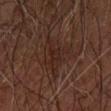body site: the left forearm
patient: male, about 70 years old
image source: ~15 mm tile from a whole-body skin photo
tile lighting: cross-polarized illumination
automated lesion analysis: a footprint of about 15 mm², an outline eccentricity of about 0.7 (0 = round, 1 = elongated), and a symmetry-axis asymmetry near 0.5; a mean CIELAB color near L≈19 a*≈14 b*≈18 and a normalized lesion–skin contrast near 5.5; a border-irregularity rating of about 6.5/10, internal color variation of about 2.5 on a 0–10 scale, and radial color variation of about 0.5; a classifier nevus-likeness of about 0/100